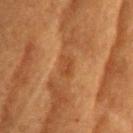- notes: catalogued during a skin exam; not biopsied
- subject: female, aged around 80
- automated lesion analysis: a border-irregularity index near 2.5/10, a color-variation rating of about 2/10, and radial color variation of about 0.5
- size: ~2.5 mm (longest diameter)
- lighting: cross-polarized
- image: ~15 mm crop, total-body skin-cancer survey
- anatomic site: the head or neck